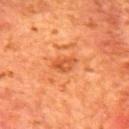The lesion was photographed on a routine skin check and not biopsied; there is no pathology result.
From the mid back.
The total-body-photography lesion software estimated an area of roughly 4 mm² and an eccentricity of roughly 0.8. The analysis additionally found a normalized lesion–skin contrast near 6. The software also gave a border-irregularity rating of about 3.5/10. It also reported a classifier nevus-likeness of about 15/100 and lesion-presence confidence of about 100/100.
Imaged with cross-polarized lighting.
Cropped from a total-body skin-imaging series; the visible field is about 15 mm.
The patient is a male roughly 65 years of age.